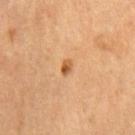The lesion was photographed on a routine skin check and not biopsied; there is no pathology result. Imaged with cross-polarized lighting. A roughly 15 mm field-of-view crop from a total-body skin photograph. Automated tile analysis of the lesion measured roughly 12 lightness units darker than nearby skin and a lesion-to-skin contrast of about 8 (normalized; higher = more distinct). And it measured border irregularity of about 2 on a 0–10 scale, a color-variation rating of about 4/10, and a peripheral color-asymmetry measure near 1.5. Approximately 2 mm at its widest. The subject is a female aged 68–72. The lesion is located on the right thigh.